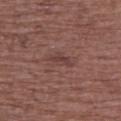| field | value |
|---|---|
| workup | imaged on a skin check; not biopsied |
| body site | the upper back |
| lesion size | ~3 mm (longest diameter) |
| patient | female, about 75 years old |
| imaging modality | total-body-photography crop, ~15 mm field of view |
| image-analysis metrics | a footprint of about 3 mm², an outline eccentricity of about 0.9 (0 = round, 1 = elongated), and a symmetry-axis asymmetry near 0.3; a lesion color around L≈39 a*≈21 b*≈21 in CIELAB and a lesion–skin lightness drop of about 7; internal color variation of about 1 on a 0–10 scale and peripheral color asymmetry of about 0.5; an automated nevus-likeness rating near 0 out of 100 and lesion-presence confidence of about 100/100 |